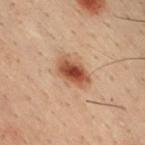biopsy_status: not biopsied; imaged during a skin examination
patient:
  sex: male
  age_approx: 30
automated_metrics:
  area_mm2_approx: 9.0
  eccentricity: 0.75
  shape_asymmetry: 0.2
  cielab_L: 42
  cielab_a: 20
  cielab_b: 27
  vs_skin_darker_L: 12.0
  vs_skin_contrast_norm: 10.0
  border_irregularity_0_10: 2.0
  color_variation_0_10: 6.5
image:
  source: total-body photography crop
  field_of_view_mm: 15
lighting: cross-polarized
lesion_size:
  long_diameter_mm_approx: 4.0
site: chest